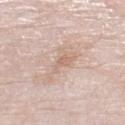This lesion was catalogued during total-body skin photography and was not selected for biopsy. From the left lower leg. The subject is a male in their 80s. Measured at roughly 6 mm in maximum diameter. This is a white-light tile. This image is a 15 mm lesion crop taken from a total-body photograph.